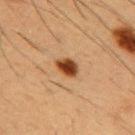Clinical impression:
Captured during whole-body skin photography for melanoma surveillance; the lesion was not biopsied.
Clinical summary:
The patient is a male aged approximately 55. A region of skin cropped from a whole-body photographic capture, roughly 15 mm wide. The lesion-visualizer software estimated roughly 17 lightness units darker than nearby skin. Located on the chest. The recorded lesion diameter is about 3 mm. Captured under cross-polarized illumination.